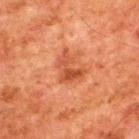automated_metrics:
  area_mm2_approx: 6.5
  eccentricity: 0.65
  shape_asymmetry: 0.5
  cielab_L: 41
  cielab_a: 27
  cielab_b: 33
  vs_skin_darker_L: 8.0
  border_irregularity_0_10: 6.0
  color_variation_0_10: 3.0
  peripheral_color_asymmetry: 1.0
image:
  source: total-body photography crop
  field_of_view_mm: 15
patient:
  sex: male
  age_approx: 80
site: upper back
lighting: cross-polarized
lesion_size:
  long_diameter_mm_approx: 3.5